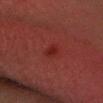* biopsy status: no biopsy performed (imaged during a skin exam)
* imaging modality: total-body-photography crop, ~15 mm field of view
* subject: male, aged approximately 30
* body site: the head or neck